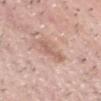- biopsy status · imaged on a skin check; not biopsied
- image · ~15 mm crop, total-body skin-cancer survey
- size · ~3 mm (longest diameter)
- illumination · white-light
- TBP lesion metrics · a mean CIELAB color near L≈60 a*≈20 b*≈26 and roughly 9 lightness units darker than nearby skin; a border-irregularity rating of about 5.5/10, a color-variation rating of about 0/10, and a peripheral color-asymmetry measure near 0
- location · the head or neck
- patient · male, approximately 50 years of age